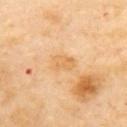  biopsy_status: not biopsied; imaged during a skin examination
  lesion_size:
    long_diameter_mm_approx: 3.0
  patient:
    sex: female
    age_approx: 60
  lighting: cross-polarized
  site: upper back
  automated_metrics:
    cielab_L: 70
    cielab_a: 20
    cielab_b: 45
    vs_skin_darker_L: 7.0
  image:
    source: total-body photography crop
    field_of_view_mm: 15